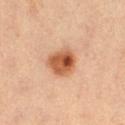{
  "biopsy_status": "not biopsied; imaged during a skin examination",
  "automated_metrics": {
    "border_irregularity_0_10": 2.0,
    "color_variation_0_10": 8.5,
    "peripheral_color_asymmetry": 3.0
  },
  "site": "right thigh",
  "lighting": "cross-polarized",
  "lesion_size": {
    "long_diameter_mm_approx": 3.5
  },
  "image": {
    "source": "total-body photography crop",
    "field_of_view_mm": 15
  },
  "patient": {
    "sex": "female",
    "age_approx": 50
  }
}